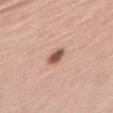biopsy_status: not biopsied; imaged during a skin examination
lesion_size:
  long_diameter_mm_approx: 3.0
lighting: white-light
image:
  source: total-body photography crop
  field_of_view_mm: 15
patient:
  sex: female
  age_approx: 65
site: chest